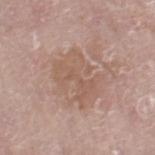No biopsy was performed on this lesion — it was imaged during a full skin examination and was not determined to be concerning. Automated image analysis of the tile measured a footprint of about 15 mm², a shape eccentricity near 0.7, and a symmetry-axis asymmetry near 0.4. The software also gave a border-irregularity index near 8/10, internal color variation of about 3 on a 0–10 scale, and radial color variation of about 1. The software also gave a classifier nevus-likeness of about 0/100 and a lesion-detection confidence of about 100/100. Measured at roughly 5.5 mm in maximum diameter. A region of skin cropped from a whole-body photographic capture, roughly 15 mm wide. This is a white-light tile. Located on the leg. A female patient, in their mid-80s or older.